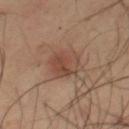| feature | finding |
|---|---|
| notes | no biopsy performed (imaged during a skin exam) |
| patient | male, aged 48 to 52 |
| acquisition | ~15 mm crop, total-body skin-cancer survey |
| automated lesion analysis | an average lesion color of about L≈43 a*≈18 b*≈26 (CIELAB) and a lesion-to-skin contrast of about 6.5 (normalized; higher = more distinct); a border-irregularity rating of about 2/10 and a peripheral color-asymmetry measure near 1.5 |
| site | the chest |